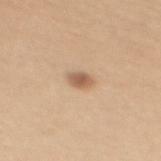| key | value |
|---|---|
| follow-up | imaged on a skin check; not biopsied |
| diameter | ~2.5 mm (longest diameter) |
| image source | 15 mm crop, total-body photography |
| anatomic site | the mid back |
| automated metrics | an eccentricity of roughly 0.75 and a shape-asymmetry score of about 0.25 (0 = symmetric) |
| tile lighting | white-light |
| patient | female, aged approximately 30 |